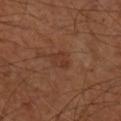Impression: Captured during whole-body skin photography for melanoma surveillance; the lesion was not biopsied.